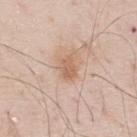Clinical impression: The lesion was photographed on a routine skin check and not biopsied; there is no pathology result. Background: A close-up tile cropped from a whole-body skin photograph, about 15 mm across. The lesion's longest dimension is about 2.5 mm. The patient is a male approximately 80 years of age. The lesion is on the back.